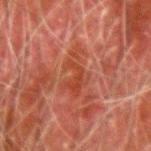Acquisition and patient details:
The tile uses cross-polarized illumination. A region of skin cropped from a whole-body photographic capture, roughly 15 mm wide. The patient is a male aged around 80. From the right forearm. The recorded lesion diameter is about 4.5 mm. An algorithmic analysis of the crop reported a mean CIELAB color near L≈35 a*≈27 b*≈29, roughly 6 lightness units darker than nearby skin, and a normalized border contrast of about 6. The software also gave internal color variation of about 1.5 on a 0–10 scale and peripheral color asymmetry of about 0.5.